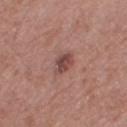Q: Is there a histopathology result?
A: catalogued during a skin exam; not biopsied
Q: How was the tile lit?
A: white-light illumination
Q: How was this image acquired?
A: ~15 mm crop, total-body skin-cancer survey
Q: How large is the lesion?
A: ~2.5 mm (longest diameter)
Q: Patient demographics?
A: female, aged around 40
Q: Where on the body is the lesion?
A: the right thigh
Q: Automated lesion metrics?
A: two-axis asymmetry of about 0.3; a mean CIELAB color near L≈45 a*≈22 b*≈22, roughly 11 lightness units darker than nearby skin, and a lesion-to-skin contrast of about 8.5 (normalized; higher = more distinct)